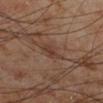Q: What lighting was used for the tile?
A: cross-polarized
Q: What is the anatomic site?
A: the left lower leg
Q: What are the patient's age and sex?
A: male, about 70 years old
Q: What kind of image is this?
A: total-body-photography crop, ~15 mm field of view
Q: Automated lesion metrics?
A: a shape eccentricity near 0.8 and a shape-asymmetry score of about 0.3 (0 = symmetric); an average lesion color of about L≈36 a*≈18 b*≈25 (CIELAB), roughly 6 lightness units darker than nearby skin, and a normalized border contrast of about 6; a detector confidence of about 100 out of 100 that the crop contains a lesion
Q: What is the lesion's diameter?
A: ≈2.5 mm